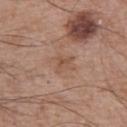workup: no biopsy performed (imaged during a skin exam)
tile lighting: white-light
image-analysis metrics: a lesion area of about 3 mm² and a shape-asymmetry score of about 0.45 (0 = symmetric); a lesion color around L≈51 a*≈20 b*≈29 in CIELAB, roughly 7 lightness units darker than nearby skin, and a normalized lesion–skin contrast near 5.5
site: the left upper arm
patient: male, aged approximately 65
acquisition: ~15 mm crop, total-body skin-cancer survey